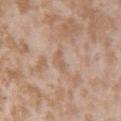This lesion was catalogued during total-body skin photography and was not selected for biopsy.
Cropped from a total-body skin-imaging series; the visible field is about 15 mm.
A female subject approximately 25 years of age.
From the left upper arm.